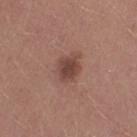workup: catalogued during a skin exam; not biopsied
tile lighting: white-light illumination
automated metrics: an average lesion color of about L≈43 a*≈21 b*≈24 (CIELAB), roughly 11 lightness units darker than nearby skin, and a normalized border contrast of about 8.5; a border-irregularity rating of about 2/10 and a within-lesion color-variation index near 2/10
location: the left thigh
acquisition: 15 mm crop, total-body photography
lesion diameter: about 3.5 mm
patient: female, roughly 20 years of age